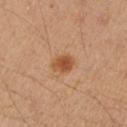The lesion was tiled from a total-body skin photograph and was not biopsied.
The patient is a female approximately 35 years of age.
From the right forearm.
The total-body-photography lesion software estimated a footprint of about 5 mm² and an outline eccentricity of about 0.5 (0 = round, 1 = elongated). And it measured a lesion–skin lightness drop of about 11 and a lesion-to-skin contrast of about 8.5 (normalized; higher = more distinct). It also reported internal color variation of about 2.5 on a 0–10 scale and radial color variation of about 1.
This is a cross-polarized tile.
A roughly 15 mm field-of-view crop from a total-body skin photograph.